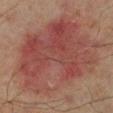  biopsy_status: not biopsied; imaged during a skin examination
  lesion_size:
    long_diameter_mm_approx: 13.0
  site: right lower leg
  patient:
    sex: male
    age_approx: 65
  image:
    source: total-body photography crop
    field_of_view_mm: 15